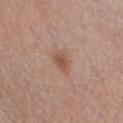Imaged during a routine full-body skin examination; the lesion was not biopsied and no histopathology is available.
Located on the chest.
An algorithmic analysis of the crop reported a footprint of about 4.5 mm² and an eccentricity of roughly 0.75.
Measured at roughly 3 mm in maximum diameter.
Captured under white-light illumination.
A male subject aged 38 to 42.
A 15 mm close-up tile from a total-body photography series done for melanoma screening.